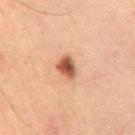biopsy_status: not biopsied; imaged during a skin examination
site: abdomen
image:
  source: total-body photography crop
  field_of_view_mm: 15
patient:
  sex: male
  age_approx: 60
lighting: cross-polarized